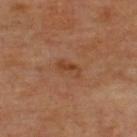Recorded during total-body skin imaging; not selected for excision or biopsy. A 15 mm close-up extracted from a 3D total-body photography capture. The tile uses cross-polarized illumination. Measured at roughly 3 mm in maximum diameter. The subject is in their mid-60s. The lesion is on the upper back. An algorithmic analysis of the crop reported an outline eccentricity of about 0.9 (0 = round, 1 = elongated) and a shape-asymmetry score of about 0.3 (0 = symmetric).